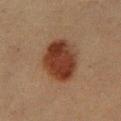Impression:
Part of a total-body skin-imaging series; this lesion was reviewed on a skin check and was not flagged for biopsy.
Context:
A close-up tile cropped from a whole-body skin photograph, about 15 mm across. On the left lower leg. Imaged with cross-polarized lighting. A male subject, aged around 45. The recorded lesion diameter is about 5.5 mm.